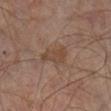Image and clinical context:
The subject is a male in their mid-60s. This image is a 15 mm lesion crop taken from a total-body photograph. The lesion is located on the right lower leg. Measured at roughly 3 mm in maximum diameter. Captured under cross-polarized illumination.